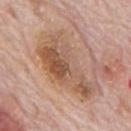biopsy status=catalogued during a skin exam; not biopsied
lighting=white-light
image source=15 mm crop, total-body photography
automated lesion analysis=a normalized border contrast of about 7.5; internal color variation of about 9 on a 0–10 scale
site=the mid back
lesion size=~9 mm (longest diameter)
patient=male, in their mid-70s